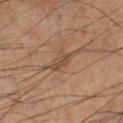biopsy status = catalogued during a skin exam; not biopsied